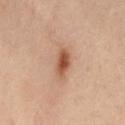Clinical impression: Recorded during total-body skin imaging; not selected for excision or biopsy. Image and clinical context: Cropped from a total-body skin-imaging series; the visible field is about 15 mm. A female patient in their mid-50s. From the abdomen.